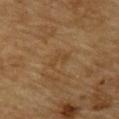follow-up: catalogued during a skin exam; not biopsied | location: the back | automated metrics: a footprint of about 2.5 mm², an outline eccentricity of about 0.85 (0 = round, 1 = elongated), and a shape-asymmetry score of about 0.55 (0 = symmetric); a lesion-detection confidence of about 100/100 | lesion diameter: ≈2.5 mm | image: ~15 mm crop, total-body skin-cancer survey | lighting: cross-polarized illumination | patient: male, in their mid- to late 80s or older.